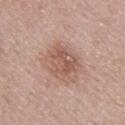Image and clinical context:
A female subject, aged approximately 50. Longest diameter approximately 5 mm. The total-body-photography lesion software estimated an eccentricity of roughly 0.5 and a shape-asymmetry score of about 0.2 (0 = symmetric). It also reported border irregularity of about 2.5 on a 0–10 scale, a color-variation rating of about 5/10, and peripheral color asymmetry of about 2. The analysis additionally found a nevus-likeness score of about 35/100 and a detector confidence of about 100 out of 100 that the crop contains a lesion. The tile uses white-light illumination. A 15 mm crop from a total-body photograph taken for skin-cancer surveillance. The lesion is on the upper back.